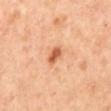Captured during whole-body skin photography for melanoma surveillance; the lesion was not biopsied.